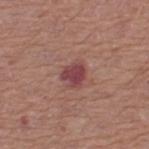| key | value |
|---|---|
| workup | imaged on a skin check; not biopsied |
| lesion diameter | ~2.5 mm (longest diameter) |
| location | the leg |
| acquisition | ~15 mm crop, total-body skin-cancer survey |
| tile lighting | white-light illumination |
| subject | female, in their 50s |